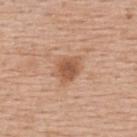Clinical impression:
Imaged during a routine full-body skin examination; the lesion was not biopsied and no histopathology is available.
Context:
A female patient aged approximately 65. A 15 mm crop from a total-body photograph taken for skin-cancer surveillance. Imaged with white-light lighting. Automated tile analysis of the lesion measured an average lesion color of about L≈55 a*≈22 b*≈33 (CIELAB) and a normalized border contrast of about 7.5. The software also gave a within-lesion color-variation index near 2.5/10 and a peripheral color-asymmetry measure near 0.5. And it measured a classifier nevus-likeness of about 80/100. The lesion is located on the back. Measured at roughly 3.5 mm in maximum diameter.